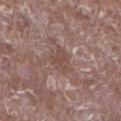No biopsy was performed on this lesion — it was imaged during a full skin examination and was not determined to be concerning. From the left lower leg. A male patient aged around 65. Imaged with white-light lighting. Automated image analysis of the tile measured a footprint of about 6 mm². The software also gave an average lesion color of about L≈47 a*≈18 b*≈22 (CIELAB), a lesion–skin lightness drop of about 7, and a normalized lesion–skin contrast near 5.5. The analysis additionally found a nevus-likeness score of about 0/100 and lesion-presence confidence of about 60/100. The lesion's longest dimension is about 3 mm. A region of skin cropped from a whole-body photographic capture, roughly 15 mm wide.